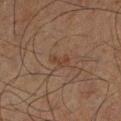Case summary:
– size · ≈2.5 mm
– image · ~15 mm tile from a whole-body skin photo
– body site · the leg
– subject · male, aged approximately 65